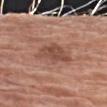Assessment: Captured during whole-body skin photography for melanoma surveillance; the lesion was not biopsied.